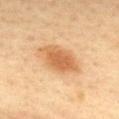No biopsy was performed on this lesion — it was imaged during a full skin examination and was not determined to be concerning.
A 15 mm close-up extracted from a 3D total-body photography capture.
From the upper back.
A female subject, about 45 years old.
Captured under cross-polarized illumination.
The lesion-visualizer software estimated a shape eccentricity near 0.8. The analysis additionally found a mean CIELAB color near L≈54 a*≈21 b*≈37, a lesion–skin lightness drop of about 11, and a normalized lesion–skin contrast near 8. The analysis additionally found a border-irregularity rating of about 2.5/10 and peripheral color asymmetry of about 1. The analysis additionally found an automated nevus-likeness rating near 100 out of 100 and lesion-presence confidence of about 100/100.